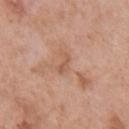<lesion>
<biopsy_status>not biopsied; imaged during a skin examination</biopsy_status>
<lighting>white-light</lighting>
<lesion_size>
  <long_diameter_mm_approx>2.5</long_diameter_mm_approx>
</lesion_size>
<automated_metrics>
  <area_mm2_approx>3.0</area_mm2_approx>
  <shape_asymmetry>0.4</shape_asymmetry>
  <cielab_L>57</cielab_L>
  <cielab_a>22</cielab_a>
  <cielab_b>31</cielab_b>
  <vs_skin_darker_L>7.0</vs_skin_darker_L>
  <border_irregularity_0_10>4.0</border_irregularity_0_10>
  <color_variation_0_10>2.0</color_variation_0_10>
  <nevus_likeness_0_100>0</nevus_likeness_0_100>
</automated_metrics>
<patient>
  <sex>male</sex>
  <age_approx>70</age_approx>
</patient>
<site>chest</site>
<image>
  <source>total-body photography crop</source>
  <field_of_view_mm>15</field_of_view_mm>
</image>
</lesion>